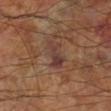biopsy_status: not biopsied; imaged during a skin examination
image:
  source: total-body photography crop
  field_of_view_mm: 15
lighting: cross-polarized
site: left lower leg
patient:
  sex: male
  age_approx: 60
lesion_size:
  long_diameter_mm_approx: 3.5